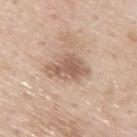Imaged during a routine full-body skin examination; the lesion was not biopsied and no histopathology is available. A male subject about 80 years old. A lesion tile, about 15 mm wide, cut from a 3D total-body photograph. The lesion is on the upper back. Captured under white-light illumination. The lesion's longest dimension is about 5 mm.